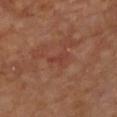Impression:
No biopsy was performed on this lesion — it was imaged during a full skin examination and was not determined to be concerning.
Clinical summary:
Located on the chest. A 15 mm crop from a total-body photograph taken for skin-cancer surveillance. The recorded lesion diameter is about 3 mm. A male patient aged 53–57.